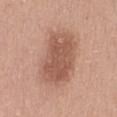biopsy_status: not biopsied; imaged during a skin examination
image:
  source: total-body photography crop
  field_of_view_mm: 15
site: lower back
patient:
  sex: female
  age_approx: 65